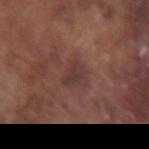Imaged with white-light lighting.
Automated tile analysis of the lesion measured a lesion area of about 3.5 mm² and a shape-asymmetry score of about 0.4 (0 = symmetric). The software also gave border irregularity of about 3.5 on a 0–10 scale.
Located on the right forearm.
Measured at roughly 2.5 mm in maximum diameter.
The subject is a male in their mid-70s.
A roughly 15 mm field-of-view crop from a total-body skin photograph.